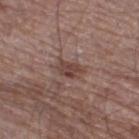subject = male, roughly 70 years of age
anatomic site = the leg
acquisition = ~15 mm tile from a whole-body skin photo